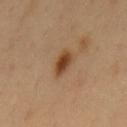No biopsy was performed on this lesion — it was imaged during a full skin examination and was not determined to be concerning. From the back. A male patient, aged approximately 50. Automated image analysis of the tile measured a lesion-detection confidence of about 100/100. A close-up tile cropped from a whole-body skin photograph, about 15 mm across.